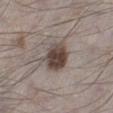Captured during whole-body skin photography for melanoma surveillance; the lesion was not biopsied.
A male subject in their 70s.
The lesion is on the left lower leg.
Cropped from a total-body skin-imaging series; the visible field is about 15 mm.
The tile uses white-light illumination.
Approximately 4 mm at its widest.